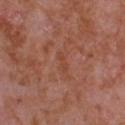Image and clinical context: A roughly 15 mm field-of-view crop from a total-body skin photograph. A male subject, approximately 65 years of age. The lesion is on the front of the torso. About 2.5 mm across.